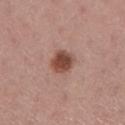Recorded during total-body skin imaging; not selected for excision or biopsy.
The subject is a female roughly 60 years of age.
The lesion is on the right lower leg.
A roughly 15 mm field-of-view crop from a total-body skin photograph.
This is a white-light tile.
The lesion's longest dimension is about 3 mm.